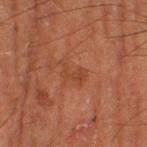Clinical impression:
Part of a total-body skin-imaging series; this lesion was reviewed on a skin check and was not flagged for biopsy.
Image and clinical context:
The lesion is on the right thigh. A 15 mm close-up extracted from a 3D total-body photography capture. Measured at roughly 3 mm in maximum diameter. The subject is a male aged 63–67.